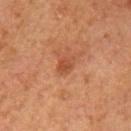Findings:
– biopsy status · catalogued during a skin exam; not biopsied
– imaging modality · ~15 mm crop, total-body skin-cancer survey
– lesion size · ~2.5 mm (longest diameter)
– subject · female, aged 58–62
– anatomic site · the arm